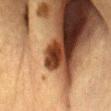Impression:
No biopsy was performed on this lesion — it was imaged during a full skin examination and was not determined to be concerning.
Context:
Imaged with cross-polarized lighting. Automated tile analysis of the lesion measured a nevus-likeness score of about 25/100 and a lesion-detection confidence of about 100/100. A female subject, aged approximately 55. Longest diameter approximately 2.5 mm. The lesion is located on the abdomen. A 15 mm close-up tile from a total-body photography series done for melanoma screening.